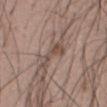follow-up = no biopsy performed (imaged during a skin exam); size = ~4.5 mm (longest diameter); body site = the front of the torso; subject = male, aged 53 to 57; image = ~15 mm tile from a whole-body skin photo.